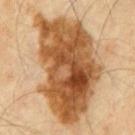Q: Was this lesion biopsied?
A: total-body-photography surveillance lesion; no biopsy
Q: Who is the patient?
A: male, approximately 60 years of age
Q: What kind of image is this?
A: total-body-photography crop, ~15 mm field of view
Q: How was the tile lit?
A: cross-polarized
Q: What is the anatomic site?
A: the abdomen